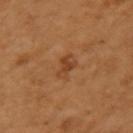Assessment:
Recorded during total-body skin imaging; not selected for excision or biopsy.
Background:
On the left upper arm. A female patient aged 53–57. A 15 mm close-up tile from a total-body photography series done for melanoma screening. The tile uses cross-polarized illumination.